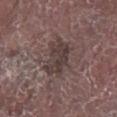Q: Is there a histopathology result?
A: catalogued during a skin exam; not biopsied
Q: How large is the lesion?
A: ~4 mm (longest diameter)
Q: Lesion location?
A: the right lower leg
Q: What lighting was used for the tile?
A: white-light illumination
Q: Who is the patient?
A: male, aged 73 to 77
Q: How was this image acquired?
A: total-body-photography crop, ~15 mm field of view